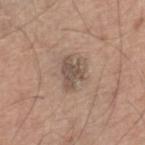Clinical impression:
Recorded during total-body skin imaging; not selected for excision or biopsy.
Clinical summary:
A 15 mm crop from a total-body photograph taken for skin-cancer surveillance. On the right lower leg. The patient is a male aged 63–67.